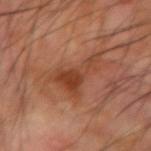The lesion was tiled from a total-body skin photograph and was not biopsied. Approximately 6.5 mm at its widest. From the left forearm. An algorithmic analysis of the crop reported an eccentricity of roughly 0.9 and two-axis asymmetry of about 0.7. It also reported a lesion color around L≈42 a*≈25 b*≈32 in CIELAB, a lesion–skin lightness drop of about 9, and a normalized lesion–skin contrast near 7.5. And it measured an automated nevus-likeness rating near 20 out of 100. Cropped from a total-body skin-imaging series; the visible field is about 15 mm. Captured under cross-polarized illumination. A male patient, aged 68–72.